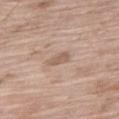Part of a total-body skin-imaging series; this lesion was reviewed on a skin check and was not flagged for biopsy.